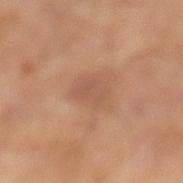Context: A male patient, aged around 50. Approximately 3 mm at its widest. Cropped from a total-body skin-imaging series; the visible field is about 15 mm. From the left lower leg.